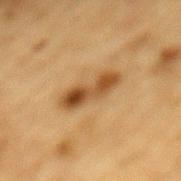Part of a total-body skin-imaging series; this lesion was reviewed on a skin check and was not flagged for biopsy. A male subject, about 85 years old or older. A 15 mm crop from a total-body photograph taken for skin-cancer surveillance. Captured under cross-polarized illumination. On the mid back.